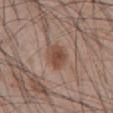No biopsy was performed on this lesion — it was imaged during a full skin examination and was not determined to be concerning. From the abdomen. A male subject aged approximately 45. Measured at roughly 3 mm in maximum diameter. Automated image analysis of the tile measured a lesion-to-skin contrast of about 8 (normalized; higher = more distinct). The software also gave a border-irregularity rating of about 2/10, internal color variation of about 2.5 on a 0–10 scale, and peripheral color asymmetry of about 1. And it measured an automated nevus-likeness rating near 90 out of 100 and a detector confidence of about 100 out of 100 that the crop contains a lesion. Captured under white-light illumination. A 15 mm close-up tile from a total-body photography series done for melanoma screening.